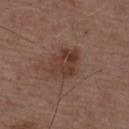Impression:
Recorded during total-body skin imaging; not selected for excision or biopsy.
Image and clinical context:
Cropped from a total-body skin-imaging series; the visible field is about 15 mm. The patient is a male aged around 50. From the back.